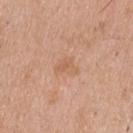The lesion was tiled from a total-body skin photograph and was not biopsied. Cropped from a total-body skin-imaging series; the visible field is about 15 mm. The lesion is located on the upper back. Automated tile analysis of the lesion measured a lesion area of about 4.5 mm² and an outline eccentricity of about 0.75 (0 = round, 1 = elongated). The analysis additionally found a mean CIELAB color near L≈61 a*≈21 b*≈34, a lesion–skin lightness drop of about 6, and a lesion-to-skin contrast of about 5 (normalized; higher = more distinct). And it measured a border-irregularity index near 4/10, a within-lesion color-variation index near 2/10, and peripheral color asymmetry of about 0.5. And it measured an automated nevus-likeness rating near 0 out of 100. Imaged with white-light lighting. The subject is a male about 40 years old. The lesion's longest dimension is about 3 mm.